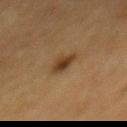workup = no biopsy performed (imaged during a skin exam) | lesion size = about 3 mm | imaging modality = ~15 mm tile from a whole-body skin photo | site = the mid back | patient = female, about 55 years old | tile lighting = cross-polarized.